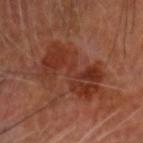Imaged during a routine full-body skin examination; the lesion was not biopsied and no histopathology is available.